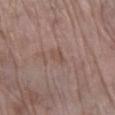biopsy_status: not biopsied; imaged during a skin examination
image:
  source: total-body photography crop
  field_of_view_mm: 15
lighting: white-light
automated_metrics:
  area_mm2_approx: 3.5
  eccentricity: 0.8
  shape_asymmetry: 0.2
  cielab_L: 50
  cielab_a: 18
  cielab_b: 23
  lesion_detection_confidence_0_100: 100
site: right lower leg
patient:
  sex: female
  age_approx: 75